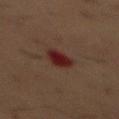workup=no biopsy performed (imaged during a skin exam).